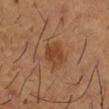  biopsy_status: not biopsied; imaged during a skin examination
  image:
    source: total-body photography crop
    field_of_view_mm: 15
  patient:
    sex: male
    age_approx: 55
  lesion_size:
    long_diameter_mm_approx: 3.0
  site: right forearm
  lighting: cross-polarized